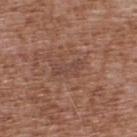{
  "biopsy_status": "not biopsied; imaged during a skin examination",
  "image": {
    "source": "total-body photography crop",
    "field_of_view_mm": 15
  },
  "patient": {
    "sex": "male",
    "age_approx": 75
  },
  "site": "upper back"
}